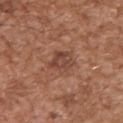Impression:
Part of a total-body skin-imaging series; this lesion was reviewed on a skin check and was not flagged for biopsy.
Context:
The patient is a male in their mid-40s. A 15 mm close-up extracted from a 3D total-body photography capture. The lesion is located on the left upper arm. Automated tile analysis of the lesion measured an average lesion color of about L≈44 a*≈22 b*≈27 (CIELAB), a lesion–skin lightness drop of about 8, and a normalized border contrast of about 7. It also reported border irregularity of about 3 on a 0–10 scale. It also reported an automated nevus-likeness rating near 0 out of 100 and a detector confidence of about 100 out of 100 that the crop contains a lesion. Longest diameter approximately 3.5 mm.